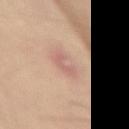Imaged during a routine full-body skin examination; the lesion was not biopsied and no histopathology is available. Imaged with white-light lighting. A male patient, aged 63–67. A roughly 15 mm field-of-view crop from a total-body skin photograph. The lesion is located on the right thigh. The total-body-photography lesion software estimated an eccentricity of roughly 0.85 and a symmetry-axis asymmetry near 0.45. The analysis additionally found a nevus-likeness score of about 0/100 and a lesion-detection confidence of about 100/100. Approximately 3 mm at its widest.